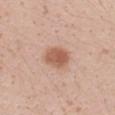Q: Was a biopsy performed?
A: no biopsy performed (imaged during a skin exam)
Q: What is the imaging modality?
A: total-body-photography crop, ~15 mm field of view
Q: What are the patient's age and sex?
A: female, aged around 20
Q: Lesion location?
A: the right forearm
Q: How large is the lesion?
A: ≈3.5 mm